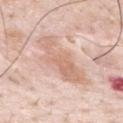The lesion was photographed on a routine skin check and not biopsied; there is no pathology result. A male subject, aged around 35. Automated tile analysis of the lesion measured an area of roughly 19 mm² and two-axis asymmetry of about 0.35. On the upper back. A lesion tile, about 15 mm wide, cut from a 3D total-body photograph. Measured at roughly 7 mm in maximum diameter.